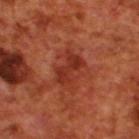Assessment: Recorded during total-body skin imaging; not selected for excision or biopsy. Background: This is a cross-polarized tile. The lesion is located on the upper back. Automated image analysis of the tile measured a within-lesion color-variation index near 4/10. It also reported an automated nevus-likeness rating near 70 out of 100. Longest diameter approximately 4 mm. The patient is a male aged around 70. A lesion tile, about 15 mm wide, cut from a 3D total-body photograph.